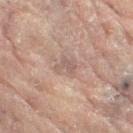Imaged during a routine full-body skin examination; the lesion was not biopsied and no histopathology is available. The lesion is on the left leg. A female patient, approximately 80 years of age. The tile uses cross-polarized illumination. Cropped from a whole-body photographic skin survey; the tile spans about 15 mm. The recorded lesion diameter is about 2.5 mm.